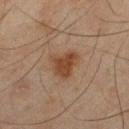automated lesion analysis: an outline eccentricity of about 0.55 (0 = round, 1 = elongated) and a shape-asymmetry score of about 0.35 (0 = symmetric) | patient: male, approximately 45 years of age | acquisition: ~15 mm crop, total-body skin-cancer survey | anatomic site: the left thigh | lesion size: ≈3.5 mm | tile lighting: cross-polarized illumination.